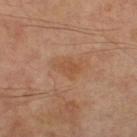Part of a total-body skin-imaging series; this lesion was reviewed on a skin check and was not flagged for biopsy. Approximately 3 mm at its widest. This is a cross-polarized tile. On the right thigh. A male patient approximately 70 years of age. Cropped from a total-body skin-imaging series; the visible field is about 15 mm. Automated tile analysis of the lesion measured border irregularity of about 3.5 on a 0–10 scale and radial color variation of about 0.5. The analysis additionally found an automated nevus-likeness rating near 0 out of 100 and a lesion-detection confidence of about 100/100.